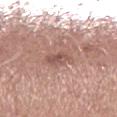follow-up=catalogued during a skin exam; not biopsied | subject=male, approximately 45 years of age | lighting=white-light illumination | lesion size=≈3 mm | automated lesion analysis=an average lesion color of about L≈53 a*≈21 b*≈24 (CIELAB), about 9 CIELAB-L* units darker than the surrounding skin, and a normalized lesion–skin contrast near 6.5; a within-lesion color-variation index near 3/10 | anatomic site=the right lower leg | acquisition=~15 mm crop, total-body skin-cancer survey.